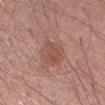The lesion was tiled from a total-body skin photograph and was not biopsied. A male subject, aged approximately 50. On the right upper arm. A 15 mm close-up tile from a total-body photography series done for melanoma screening.